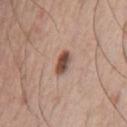Impression:
Part of a total-body skin-imaging series; this lesion was reviewed on a skin check and was not flagged for biopsy.
Context:
A male subject, aged approximately 75. The lesion is located on the front of the torso. An algorithmic analysis of the crop reported a lesion area of about 4.5 mm². The software also gave roughly 15 lightness units darker than nearby skin and a normalized lesion–skin contrast near 10.5. It also reported border irregularity of about 1.5 on a 0–10 scale, a within-lesion color-variation index near 5/10, and peripheral color asymmetry of about 1.5. About 3 mm across. The tile uses white-light illumination. This image is a 15 mm lesion crop taken from a total-body photograph.